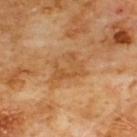• follow-up · catalogued during a skin exam; not biopsied
• acquisition · ~15 mm crop, total-body skin-cancer survey
• automated lesion analysis · an area of roughly 7.5 mm²; a lesion color around L≈51 a*≈22 b*≈40 in CIELAB, a lesion–skin lightness drop of about 7, and a normalized lesion–skin contrast near 5.5; border irregularity of about 6.5 on a 0–10 scale, internal color variation of about 3 on a 0–10 scale, and radial color variation of about 1
• patient · male, approximately 60 years of age
• location · the chest